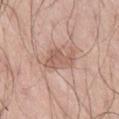A 15 mm close-up tile from a total-body photography series done for melanoma screening. Measured at roughly 4.5 mm in maximum diameter. The lesion is located on the right thigh. A male subject, about 70 years old.